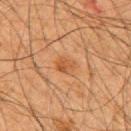A lesion tile, about 15 mm wide, cut from a 3D total-body photograph.
A male subject, aged around 65.
Measured at roughly 2.5 mm in maximum diameter.
Imaged with cross-polarized lighting.
The lesion is located on the upper back.